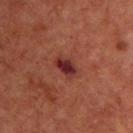<lesion>
  <lesion_size>
    <long_diameter_mm_approx>3.0</long_diameter_mm_approx>
  </lesion_size>
  <site>chest</site>
  <patient>
    <sex>male</sex>
    <age_approx>65</age_approx>
  </patient>
  <lighting>cross-polarized</lighting>
  <image>
    <source>total-body photography crop</source>
    <field_of_view_mm>15</field_of_view_mm>
  </image>
</lesion>